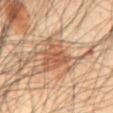This lesion was catalogued during total-body skin photography and was not selected for biopsy. Cropped from a total-body skin-imaging series; the visible field is about 15 mm. The lesion is located on the abdomen. The subject is a male roughly 45 years of age. Imaged with cross-polarized lighting. Automated image analysis of the tile measured an average lesion color of about L≈55 a*≈20 b*≈32 (CIELAB), roughly 10 lightness units darker than nearby skin, and a normalized border contrast of about 7. It also reported a nevus-likeness score of about 55/100 and a detector confidence of about 100 out of 100 that the crop contains a lesion. About 5 mm across.